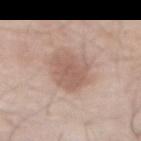Impression: Captured during whole-body skin photography for melanoma surveillance; the lesion was not biopsied. Background: A male subject about 80 years old. The lesion is located on the abdomen. Captured under white-light illumination. About 5.5 mm across. A 15 mm crop from a total-body photograph taken for skin-cancer surveillance.